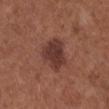| field | value |
|---|---|
| biopsy status | no biopsy performed (imaged during a skin exam) |